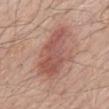<lesion>
  <biopsy_status>not biopsied; imaged during a skin examination</biopsy_status>
  <lighting>white-light</lighting>
  <image>
    <source>total-body photography crop</source>
    <field_of_view_mm>15</field_of_view_mm>
  </image>
  <lesion_size>
    <long_diameter_mm_approx>6.5</long_diameter_mm_approx>
  </lesion_size>
  <patient>
    <sex>male</sex>
    <age_approx>70</age_approx>
  </patient>
  <automated_metrics>
    <cielab_L>54</cielab_L>
    <cielab_a>23</cielab_a>
    <cielab_b>26</cielab_b>
    <vs_skin_darker_L>10.0</vs_skin_darker_L>
  </automated_metrics>
  <site>back</site>
</lesion>